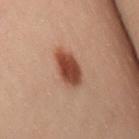biopsy status: imaged on a skin check; not biopsied
size: ~4.5 mm (longest diameter)
patient: female, aged 28–32
image: ~15 mm crop, total-body skin-cancer survey
site: the left leg
tile lighting: cross-polarized